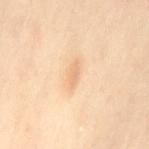Recorded during total-body skin imaging; not selected for excision or biopsy.
On the left thigh.
A 15 mm close-up extracted from a 3D total-body photography capture.
A female patient, aged 53 to 57.
This is a cross-polarized tile.